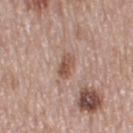No biopsy was performed on this lesion — it was imaged during a full skin examination and was not determined to be concerning.
A female patient, aged approximately 50.
Cropped from a total-body skin-imaging series; the visible field is about 15 mm.
From the right thigh.